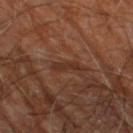follow-up: catalogued during a skin exam; not biopsied
tile lighting: cross-polarized illumination
lesion size: ≈3.5 mm
body site: the leg
imaging modality: ~15 mm tile from a whole-body skin photo
subject: male, about 60 years old
automated metrics: a mean CIELAB color near L≈30 a*≈20 b*≈26 and a normalized lesion–skin contrast near 6; border irregularity of about 4.5 on a 0–10 scale and internal color variation of about 0 on a 0–10 scale; a classifier nevus-likeness of about 0/100 and a detector confidence of about 65 out of 100 that the crop contains a lesion